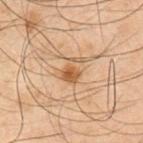Impression:
Captured during whole-body skin photography for melanoma surveillance; the lesion was not biopsied.
Clinical summary:
The tile uses cross-polarized illumination. The patient is a male in their 50s. A roughly 15 mm field-of-view crop from a total-body skin photograph. Measured at roughly 3.5 mm in maximum diameter. An algorithmic analysis of the crop reported a lesion color around L≈45 a*≈15 b*≈30 in CIELAB, roughly 8 lightness units darker than nearby skin, and a lesion-to-skin contrast of about 7 (normalized; higher = more distinct). The lesion is on the arm.